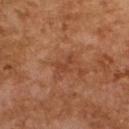Q: Was a biopsy performed?
A: catalogued during a skin exam; not biopsied
Q: Lesion size?
A: about 3 mm
Q: What did automated image analysis measure?
A: border irregularity of about 5.5 on a 0–10 scale and peripheral color asymmetry of about 0
Q: How was this image acquired?
A: 15 mm crop, total-body photography
Q: Where on the body is the lesion?
A: the upper back
Q: Patient demographics?
A: female, aged around 60
Q: How was the tile lit?
A: cross-polarized illumination